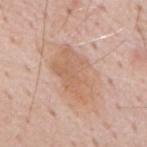biopsy status: no biopsy performed (imaged during a skin exam)
lesion diameter: ≈6.5 mm
lighting: white-light illumination
patient: male, aged around 60
automated metrics: a lesion color around L≈63 a*≈20 b*≈31 in CIELAB, roughly 8 lightness units darker than nearby skin, and a normalized lesion–skin contrast near 6; a color-variation rating of about 3/10 and peripheral color asymmetry of about 1; a classifier nevus-likeness of about 0/100 and a lesion-detection confidence of about 100/100
image source: ~15 mm crop, total-body skin-cancer survey
anatomic site: the mid back